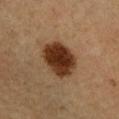Assessment:
The lesion was photographed on a routine skin check and not biopsied; there is no pathology result.
Clinical summary:
A 15 mm close-up tile from a total-body photography series done for melanoma screening. Approximately 5 mm at its widest. The patient is a female aged 43–47. The lesion is on the right forearm. The tile uses cross-polarized illumination. The total-body-photography lesion software estimated lesion-presence confidence of about 100/100.